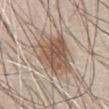{
  "biopsy_status": "not biopsied; imaged during a skin examination",
  "patient": {
    "sex": "male",
    "age_approx": 65
  },
  "image": {
    "source": "total-body photography crop",
    "field_of_view_mm": 15
  },
  "site": "front of the torso"
}